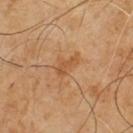{
  "biopsy_status": "not biopsied; imaged during a skin examination",
  "image": {
    "source": "total-body photography crop",
    "field_of_view_mm": 15
  },
  "patient": {
    "sex": "male",
    "age_approx": 65
  }
}